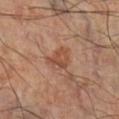Captured during whole-body skin photography for melanoma surveillance; the lesion was not biopsied. This is a cross-polarized tile. The total-body-photography lesion software estimated a lesion color around L≈46 a*≈22 b*≈30 in CIELAB, roughly 8 lightness units darker than nearby skin, and a lesion-to-skin contrast of about 6.5 (normalized; higher = more distinct). It also reported a classifier nevus-likeness of about 0/100 and a lesion-detection confidence of about 100/100. The subject is a male about 60 years old. A 15 mm crop from a total-body photograph taken for skin-cancer surveillance. The recorded lesion diameter is about 3 mm. The lesion is located on the right lower leg.